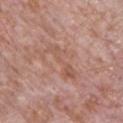The lesion was tiled from a total-body skin photograph and was not biopsied.
Longest diameter approximately 5.5 mm.
From the chest.
This image is a 15 mm lesion crop taken from a total-body photograph.
A male subject about 70 years old.
The total-body-photography lesion software estimated a footprint of about 8 mm² and an eccentricity of roughly 0.9. The software also gave a nevus-likeness score of about 0/100 and lesion-presence confidence of about 95/100.
Captured under white-light illumination.